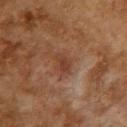Impression: No biopsy was performed on this lesion — it was imaged during a full skin examination and was not determined to be concerning. Context: A female patient, in their 60s. A close-up tile cropped from a whole-body skin photograph, about 15 mm across. Located on the upper back. Measured at roughly 2.5 mm in maximum diameter. Automated image analysis of the tile measured an automated nevus-likeness rating near 0 out of 100 and a detector confidence of about 100 out of 100 that the crop contains a lesion. The tile uses cross-polarized illumination.